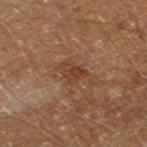Captured during whole-body skin photography for melanoma surveillance; the lesion was not biopsied.
Approximately 3 mm at its widest.
This image is a 15 mm lesion crop taken from a total-body photograph.
A male patient approximately 60 years of age.
On the leg.